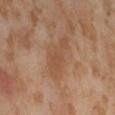Q: Was this lesion biopsied?
A: total-body-photography surveillance lesion; no biopsy
Q: Illumination type?
A: cross-polarized illumination
Q: What is the anatomic site?
A: the abdomen
Q: What is the lesion's diameter?
A: ≈5.5 mm
Q: What kind of image is this?
A: ~15 mm tile from a whole-body skin photo
Q: Patient demographics?
A: female, aged approximately 55